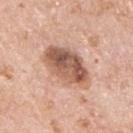biopsy status — imaged on a skin check; not biopsied
location — the chest
imaging modality — ~15 mm tile from a whole-body skin photo
patient — male, aged approximately 70
lighting — white-light illumination